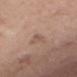location: the chest
acquisition: 15 mm crop, total-body photography
size: about 2.5 mm
subject: female, approximately 40 years of age